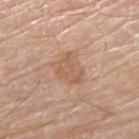Clinical impression: The lesion was photographed on a routine skin check and not biopsied; there is no pathology result. Acquisition and patient details: Captured under white-light illumination. A male subject, aged 78 to 82. Approximately 4 mm at its widest. The lesion-visualizer software estimated an outline eccentricity of about 0.6 (0 = round, 1 = elongated) and two-axis asymmetry of about 0.3. The software also gave a classifier nevus-likeness of about 10/100 and a detector confidence of about 100 out of 100 that the crop contains a lesion. A roughly 15 mm field-of-view crop from a total-body skin photograph. The lesion is located on the left arm.